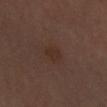{"biopsy_status": "not biopsied; imaged during a skin examination", "image": {"source": "total-body photography crop", "field_of_view_mm": 15}, "lighting": "cross-polarized", "patient": {"sex": "male", "age_approx": 65}, "site": "left forearm", "automated_metrics": {"border_irregularity_0_10": 2.5, "peripheral_color_asymmetry": 0.5}}